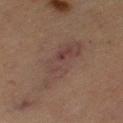Q: What is the lesion's diameter?
A: about 9 mm
Q: How was this image acquired?
A: 15 mm crop, total-body photography
Q: Where on the body is the lesion?
A: the left thigh
Q: Patient demographics?
A: female, approximately 70 years of age